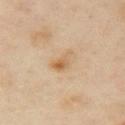biopsy_status: not biopsied; imaged during a skin examination
patient:
  sex: female
  age_approx: 40
image:
  source: total-body photography crop
  field_of_view_mm: 15
lighting: cross-polarized
lesion_size:
  long_diameter_mm_approx: 3.0
site: left arm
automated_metrics:
  eccentricity: 0.85
  shape_asymmetry: 0.45
  border_irregularity_0_10: 4.5
  color_variation_0_10: 4.5
  nevus_likeness_0_100: 10
  lesion_detection_confidence_0_100: 100